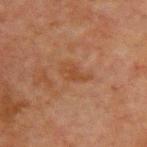Q: Was this lesion biopsied?
A: no biopsy performed (imaged during a skin exam)
Q: What is the lesion's diameter?
A: ≈3.5 mm
Q: What lighting was used for the tile?
A: cross-polarized illumination
Q: Lesion location?
A: the chest
Q: What are the patient's age and sex?
A: male, in their mid- to late 60s
Q: Automated lesion metrics?
A: a lesion color around L≈37 a*≈19 b*≈29 in CIELAB, about 5 CIELAB-L* units darker than the surrounding skin, and a lesion-to-skin contrast of about 6 (normalized; higher = more distinct)
Q: How was this image acquired?
A: total-body-photography crop, ~15 mm field of view